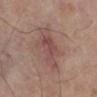Clinical impression:
This lesion was catalogued during total-body skin photography and was not selected for biopsy.
Background:
This image is a 15 mm lesion crop taken from a total-body photograph. The lesion is located on the right lower leg. The patient is a male aged 68–72. The recorded lesion diameter is about 5.5 mm.